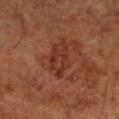This lesion was catalogued during total-body skin photography and was not selected for biopsy.
Captured under cross-polarized illumination.
The recorded lesion diameter is about 4.5 mm.
Located on the left lower leg.
A male patient, aged approximately 65.
Cropped from a whole-body photographic skin survey; the tile spans about 15 mm.